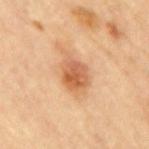Q: Was a biopsy performed?
A: catalogued during a skin exam; not biopsied
Q: Illumination type?
A: cross-polarized
Q: How large is the lesion?
A: ~5 mm (longest diameter)
Q: What did automated image analysis measure?
A: a normalized border contrast of about 7.5; an automated nevus-likeness rating near 70 out of 100 and a lesion-detection confidence of about 100/100
Q: What are the patient's age and sex?
A: male, roughly 85 years of age or older
Q: Where on the body is the lesion?
A: the back
Q: What kind of image is this?
A: ~15 mm tile from a whole-body skin photo